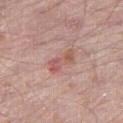Assessment: Recorded during total-body skin imaging; not selected for excision or biopsy. Acquisition and patient details: About 4 mm across. A male subject, in their mid- to late 60s. Captured under white-light illumination. Cropped from a whole-body photographic skin survey; the tile spans about 15 mm. Automated tile analysis of the lesion measured a border-irregularity index near 3.5/10, a color-variation rating of about 5.5/10, and radial color variation of about 1.5. And it measured a classifier nevus-likeness of about 0/100. Located on the right thigh.